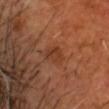Part of a total-body skin-imaging series; this lesion was reviewed on a skin check and was not flagged for biopsy. On the head or neck. Cropped from a total-body skin-imaging series; the visible field is about 15 mm. Imaged with cross-polarized lighting. The subject is a male aged approximately 50. The lesion-visualizer software estimated a classifier nevus-likeness of about 0/100.